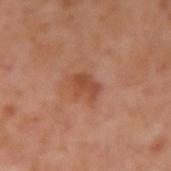{"biopsy_status": "not biopsied; imaged during a skin examination", "lesion_size": {"long_diameter_mm_approx": 3.0}, "image": {"source": "total-body photography crop", "field_of_view_mm": 15}, "site": "arm", "patient": {"sex": "male", "age_approx": 55}}